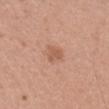<lesion>
<biopsy_status>not biopsied; imaged during a skin examination</biopsy_status>
<patient>
  <sex>female</sex>
  <age_approx>40</age_approx>
</patient>
<automated_metrics>
  <cielab_L>57</cielab_L>
  <cielab_a>23</cielab_a>
  <cielab_b>32</cielab_b>
  <vs_skin_darker_L>8.0</vs_skin_darker_L>
  <vs_skin_contrast_norm>5.5</vs_skin_contrast_norm>
  <color_variation_0_10>1.0</color_variation_0_10>
  <peripheral_color_asymmetry>0.5</peripheral_color_asymmetry>
  <nevus_likeness_0_100>30</nevus_likeness_0_100>
  <lesion_detection_confidence_0_100>100</lesion_detection_confidence_0_100>
</automated_metrics>
<site>head or neck</site>
<lighting>white-light</lighting>
<lesion_size>
  <long_diameter_mm_approx>2.0</long_diameter_mm_approx>
</lesion_size>
<image>
  <source>total-body photography crop</source>
  <field_of_view_mm>15</field_of_view_mm>
</image>
</lesion>